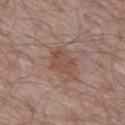Findings:
• notes: catalogued during a skin exam; not biopsied
• lighting: white-light illumination
• acquisition: total-body-photography crop, ~15 mm field of view
• patient: male, in their mid-60s
• lesion size: about 3.5 mm
• TBP lesion metrics: two-axis asymmetry of about 0.25; a nevus-likeness score of about 15/100 and a detector confidence of about 100 out of 100 that the crop contains a lesion
• location: the left thigh